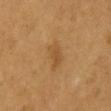| field | value |
|---|---|
| workup | total-body-photography surveillance lesion; no biopsy |
| illumination | cross-polarized illumination |
| imaging modality | ~15 mm tile from a whole-body skin photo |
| subject | male, roughly 55 years of age |
| image-analysis metrics | a mean CIELAB color near L≈44 a*≈17 b*≈36, roughly 6 lightness units darker than nearby skin, and a lesion-to-skin contrast of about 5.5 (normalized; higher = more distinct) |
| diameter | about 3.5 mm |
| anatomic site | the mid back |